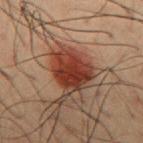biopsy status: total-body-photography surveillance lesion; no biopsy | site: the chest | patient: male, aged 48 to 52 | diameter: ≈5.5 mm | image source: ~15 mm crop, total-body skin-cancer survey | illumination: cross-polarized.